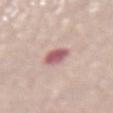Findings:
– biopsy status · imaged on a skin check; not biopsied
– lesion size · ≈3 mm
– illumination · white-light
– anatomic site · the lower back
– subject · female, aged 63 to 67
– image source · ~15 mm tile from a whole-body skin photo
– image-analysis metrics · a footprint of about 5.5 mm² and two-axis asymmetry of about 0.1; a mean CIELAB color near L≈58 a*≈26 b*≈19, about 15 CIELAB-L* units darker than the surrounding skin, and a normalized border contrast of about 9.5